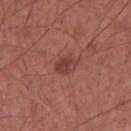Findings:
• notes — total-body-photography surveillance lesion; no biopsy
• illumination — white-light
• patient — male, approximately 65 years of age
• image source — ~15 mm crop, total-body skin-cancer survey
• size — ~2.5 mm (longest diameter)
• automated lesion analysis — an area of roughly 4 mm², an outline eccentricity of about 0.7 (0 = round, 1 = elongated), and a symmetry-axis asymmetry near 0.2; a border-irregularity rating of about 1.5/10, internal color variation of about 2.5 on a 0–10 scale, and a peripheral color-asymmetry measure near 0.5; a nevus-likeness score of about 85/100 and a lesion-detection confidence of about 100/100
• body site — the chest